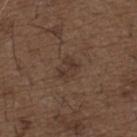The lesion was photographed on a routine skin check and not biopsied; there is no pathology result.
From the back.
Imaged with white-light lighting.
The subject is a male in their 50s.
A 15 mm close-up extracted from a 3D total-body photography capture.
Measured at roughly 2.5 mm in maximum diameter.